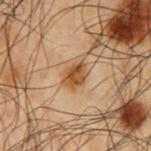workup: total-body-photography surveillance lesion; no biopsy
lighting: cross-polarized illumination
site: the right upper arm
patient: male, aged around 50
image: total-body-photography crop, ~15 mm field of view
automated lesion analysis: roughly 10 lightness units darker than nearby skin; a lesion-detection confidence of about 100/100
diameter: ~3 mm (longest diameter)